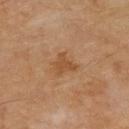Q: Who is the patient?
A: male, aged approximately 55
Q: What kind of image is this?
A: ~15 mm crop, total-body skin-cancer survey
Q: Lesion location?
A: the upper back